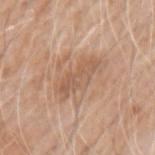• notes · total-body-photography surveillance lesion; no biopsy
• body site · the left upper arm
• subject · male, aged 58–62
• image · 15 mm crop, total-body photography